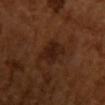| field | value |
|---|---|
| notes | imaged on a skin check; not biopsied |
| illumination | cross-polarized |
| subject | female, aged approximately 55 |
| location | the chest |
| acquisition | ~15 mm crop, total-body skin-cancer survey |